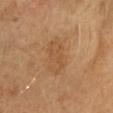Captured during whole-body skin photography for melanoma surveillance; the lesion was not biopsied. Imaged with cross-polarized lighting. A female patient, aged 53 to 57. The lesion is on the head or neck. About 5.5 mm across. A region of skin cropped from a whole-body photographic capture, roughly 15 mm wide.